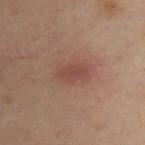{"biopsy_status": "not biopsied; imaged during a skin examination", "patient": {"sex": "male", "age_approx": 40}, "lighting": "cross-polarized", "image": {"source": "total-body photography crop", "field_of_view_mm": 15}, "lesion_size": {"long_diameter_mm_approx": 4.0}, "site": "chest"}